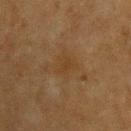<lesion>
<biopsy_status>not biopsied; imaged during a skin examination</biopsy_status>
<automated_metrics>
  <area_mm2_approx>4.0</area_mm2_approx>
  <eccentricity>0.65</eccentricity>
  <shape_asymmetry>0.3</shape_asymmetry>
  <vs_skin_darker_L>4.0</vs_skin_darker_L>
  <vs_skin_contrast_norm>5.0</vs_skin_contrast_norm>
</automated_metrics>
<site>upper back</site>
<lighting>cross-polarized</lighting>
<patient>
  <sex>male</sex>
  <age_approx>75</age_approx>
</patient>
<image>
  <source>total-body photography crop</source>
  <field_of_view_mm>15</field_of_view_mm>
</image>
</lesion>